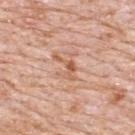{"patient": {"sex": "male", "age_approx": 80}, "image": {"source": "total-body photography crop", "field_of_view_mm": 15}, "lighting": "white-light", "site": "upper back", "lesion_size": {"long_diameter_mm_approx": 3.5}}